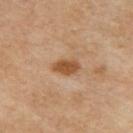  biopsy_status: not biopsied; imaged during a skin examination
  patient:
    sex: female
    age_approx: 65
  image:
    source: total-body photography crop
    field_of_view_mm: 15
  site: upper back